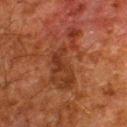Recorded during total-body skin imaging; not selected for excision or biopsy.
Longest diameter approximately 7 mm.
This is a cross-polarized tile.
The subject is a male aged 58–62.
The lesion-visualizer software estimated a shape eccentricity near 0.85 and a shape-asymmetry score of about 0.6 (0 = symmetric). It also reported border irregularity of about 9 on a 0–10 scale, a color-variation rating of about 3/10, and peripheral color asymmetry of about 1.
A 15 mm crop from a total-body photograph taken for skin-cancer surveillance.
The lesion is on the back.